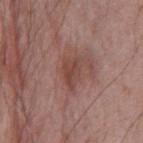Captured during whole-body skin photography for melanoma surveillance; the lesion was not biopsied.